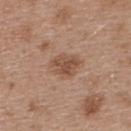| field | value |
|---|---|
| workup | catalogued during a skin exam; not biopsied |
| illumination | white-light illumination |
| image-analysis metrics | a shape eccentricity near 0.55 and a shape-asymmetry score of about 0.3 (0 = symmetric); a mean CIELAB color near L≈50 a*≈20 b*≈29 and a normalized border contrast of about 7; a border-irregularity index near 3/10, internal color variation of about 2.5 on a 0–10 scale, and a peripheral color-asymmetry measure near 1; a classifier nevus-likeness of about 25/100 and lesion-presence confidence of about 100/100 |
| location | the back |
| imaging modality | 15 mm crop, total-body photography |
| patient | female, in their 40s |